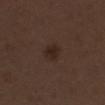The lesion was tiled from a total-body skin photograph and was not biopsied. The lesion's longest dimension is about 3 mm. The lesion is located on the head or neck. A roughly 15 mm field-of-view crop from a total-body skin photograph. A female subject, about 50 years old.